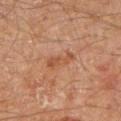The total-body-photography lesion software estimated an area of roughly 4 mm², a shape eccentricity near 0.9, and two-axis asymmetry of about 0.4. The analysis additionally found a mean CIELAB color near L≈44 a*≈21 b*≈30, roughly 7 lightness units darker than nearby skin, and a normalized lesion–skin contrast near 6.
A male patient, roughly 45 years of age.
A roughly 15 mm field-of-view crop from a total-body skin photograph.
Captured under cross-polarized illumination.
Located on the arm.